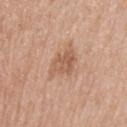Impression: Recorded during total-body skin imaging; not selected for excision or biopsy. Background: The patient is a male about 50 years old. From the back. A roughly 15 mm field-of-view crop from a total-body skin photograph.